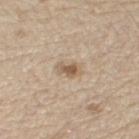The lesion was tiled from a total-body skin photograph and was not biopsied.
On the left thigh.
A male subject, about 70 years old.
A close-up tile cropped from a whole-body skin photograph, about 15 mm across.
Automated tile analysis of the lesion measured an area of roughly 4 mm² and an outline eccentricity of about 0.8 (0 = round, 1 = elongated). It also reported a mean CIELAB color near L≈58 a*≈14 b*≈31, about 11 CIELAB-L* units darker than the surrounding skin, and a normalized border contrast of about 7.5. It also reported a within-lesion color-variation index near 2/10 and peripheral color asymmetry of about 0.5.